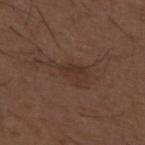Recorded during total-body skin imaging; not selected for excision or biopsy.
A roughly 15 mm field-of-view crop from a total-body skin photograph.
A male subject aged 48 to 52.
Measured at roughly 4 mm in maximum diameter.
Captured under white-light illumination.
Located on the upper back.